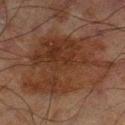Case summary:
• site: the left lower leg
• acquisition: 15 mm crop, total-body photography
• subject: male, aged 68 to 72